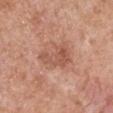lesion diameter = about 5 mm
automated lesion analysis = an area of roughly 11 mm², an eccentricity of roughly 0.75, and two-axis asymmetry of about 0.35; an average lesion color of about L≈55 a*≈23 b*≈30 (CIELAB) and a normalized border contrast of about 6; border irregularity of about 4.5 on a 0–10 scale, a color-variation rating of about 4/10, and a peripheral color-asymmetry measure near 1
location = the left upper arm
tile lighting = white-light
acquisition = 15 mm crop, total-body photography
subject = male, aged 63 to 67